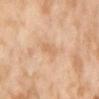No biopsy was performed on this lesion — it was imaged during a full skin examination and was not determined to be concerning. A 15 mm close-up extracted from a 3D total-body photography capture. A female subject, approximately 55 years of age. Longest diameter approximately 3 mm. The lesion-visualizer software estimated a lesion area of about 3 mm², a shape eccentricity near 0.9, and two-axis asymmetry of about 0.2. The analysis additionally found a lesion color around L≈67 a*≈21 b*≈36 in CIELAB. It also reported a nevus-likeness score of about 0/100 and lesion-presence confidence of about 100/100. The lesion is on the abdomen. Imaged with cross-polarized lighting.